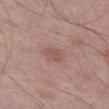{
  "biopsy_status": "not biopsied; imaged during a skin examination",
  "patient": {
    "sex": "male",
    "age_approx": 55
  },
  "site": "left thigh",
  "image": {
    "source": "total-body photography crop",
    "field_of_view_mm": 15
  }
}